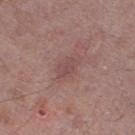Impression:
The lesion was photographed on a routine skin check and not biopsied; there is no pathology result.
Clinical summary:
Approximately 4 mm at its widest. The tile uses white-light illumination. A male patient aged approximately 50. A roughly 15 mm field-of-view crop from a total-body skin photograph. Located on the left thigh.